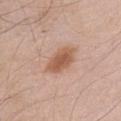Part of a total-body skin-imaging series; this lesion was reviewed on a skin check and was not flagged for biopsy.
Imaged with white-light lighting.
A male patient, aged 43 to 47.
Longest diameter approximately 4.5 mm.
Located on the chest.
This image is a 15 mm lesion crop taken from a total-body photograph.
The total-body-photography lesion software estimated a footprint of about 9 mm², an outline eccentricity of about 0.85 (0 = round, 1 = elongated), and a symmetry-axis asymmetry near 0.2. It also reported a lesion color around L≈57 a*≈21 b*≈31 in CIELAB, a lesion–skin lightness drop of about 11, and a normalized lesion–skin contrast near 8.